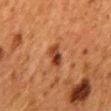Notes:
– biopsy status · total-body-photography surveillance lesion; no biopsy
– image source · ~15 mm crop, total-body skin-cancer survey
– body site · the mid back
– lesion diameter · about 3 mm
– patient · female, aged 48 to 52